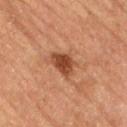Findings:
– workup · total-body-photography surveillance lesion; no biopsy
– site · the lower back
– patient · male, aged around 85 or older
– lighting · cross-polarized illumination
– image-analysis metrics · roughly 12 lightness units darker than nearby skin and a normalized border contrast of about 9.5; a border-irregularity index near 2.5/10 and a peripheral color-asymmetry measure near 1.5
– image source · ~15 mm crop, total-body skin-cancer survey